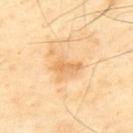biopsy status: no biopsy performed (imaged during a skin exam) | site: the mid back | automated lesion analysis: a footprint of about 2.5 mm², an outline eccentricity of about 0.95 (0 = round, 1 = elongated), and a shape-asymmetry score of about 0.45 (0 = symmetric); a mean CIELAB color near L≈72 a*≈22 b*≈46, a lesion–skin lightness drop of about 9, and a normalized lesion–skin contrast near 6; border irregularity of about 5 on a 0–10 scale and peripheral color asymmetry of about 0; a classifier nevus-likeness of about 0/100 | patient: male, aged around 65 | image source: ~15 mm crop, total-body skin-cancer survey.